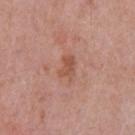No biopsy was performed on this lesion — it was imaged during a full skin examination and was not determined to be concerning. A male patient aged approximately 50. Automated image analysis of the tile measured border irregularity of about 3 on a 0–10 scale, a within-lesion color-variation index near 1.5/10, and radial color variation of about 0.5. It also reported a classifier nevus-likeness of about 0/100 and lesion-presence confidence of about 100/100. A 15 mm crop from a total-body photograph taken for skin-cancer surveillance. Located on the chest. Approximately 3 mm at its widest. Captured under white-light illumination.